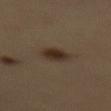Findings:
– biopsy status — imaged on a skin check; not biopsied
– body site — the right thigh
– lesion size — ≈3.5 mm
– TBP lesion metrics — an area of roughly 6 mm² and an eccentricity of roughly 0.7
– illumination — cross-polarized illumination
– subject — male, about 50 years old
– image — ~15 mm crop, total-body skin-cancer survey